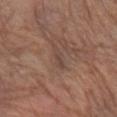{"biopsy_status": "not biopsied; imaged during a skin examination", "automated_metrics": {"area_mm2_approx": 2.5, "cielab_L": 44, "cielab_a": 17, "cielab_b": 24, "vs_skin_darker_L": 6.0, "vs_skin_contrast_norm": 5.0, "border_irregularity_0_10": 4.0, "color_variation_0_10": 0.0, "peripheral_color_asymmetry": 0.0, "nevus_likeness_0_100": 0, "lesion_detection_confidence_0_100": 50}, "image": {"source": "total-body photography crop", "field_of_view_mm": 15}, "patient": {"sex": "female", "age_approx": 65}, "site": "left forearm", "lighting": "white-light"}